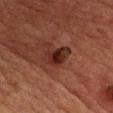Recorded during total-body skin imaging; not selected for excision or biopsy. A 15 mm crop from a total-body photograph taken for skin-cancer surveillance. The tile uses cross-polarized illumination. The subject is a male aged 63–67. Automated image analysis of the tile measured an area of roughly 7 mm² and a shape eccentricity near 0.7. The software also gave a mean CIELAB color near L≈22 a*≈20 b*≈21, about 9 CIELAB-L* units darker than the surrounding skin, and a normalized lesion–skin contrast near 10. The lesion is located on the upper back.